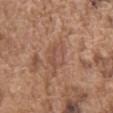Assessment: Part of a total-body skin-imaging series; this lesion was reviewed on a skin check and was not flagged for biopsy. Image and clinical context: Imaged with white-light lighting. A 15 mm crop from a total-body photograph taken for skin-cancer surveillance. A male patient, about 75 years old. From the front of the torso. Longest diameter approximately 4.5 mm.